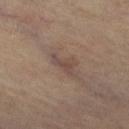notes = imaged on a skin check; not biopsied | lesion diameter = ≈3 mm | patient = female, aged approximately 65 | anatomic site = the leg | lighting = cross-polarized | image source = total-body-photography crop, ~15 mm field of view.